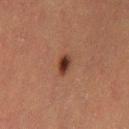Imaged during a routine full-body skin examination; the lesion was not biopsied and no histopathology is available.
A female patient aged around 55.
The lesion-visualizer software estimated an area of roughly 3.5 mm², an outline eccentricity of about 0.8 (0 = round, 1 = elongated), and two-axis asymmetry of about 0.35. The analysis additionally found a classifier nevus-likeness of about 100/100.
On the left thigh.
A region of skin cropped from a whole-body photographic capture, roughly 15 mm wide.
The lesion's longest dimension is about 2.5 mm.